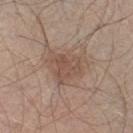Impression:
Recorded during total-body skin imaging; not selected for excision or biopsy.
Context:
A 15 mm close-up tile from a total-body photography series done for melanoma screening. Imaged with white-light lighting. The subject is a male aged approximately 60. On the leg.